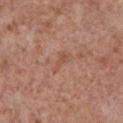The lesion was tiled from a total-body skin photograph and was not biopsied. The lesion-visualizer software estimated an eccentricity of roughly 0.95 and a shape-asymmetry score of about 0.45 (0 = symmetric). It also reported a mean CIELAB color near L≈52 a*≈22 b*≈31 and a lesion-to-skin contrast of about 5.5 (normalized; higher = more distinct). The software also gave a border-irregularity rating of about 5.5/10, internal color variation of about 0.5 on a 0–10 scale, and radial color variation of about 0. The software also gave a classifier nevus-likeness of about 0/100 and lesion-presence confidence of about 100/100. About 3 mm across. A 15 mm crop from a total-body photograph taken for skin-cancer surveillance. From the front of the torso. The subject is a male aged approximately 65. Imaged with white-light lighting.